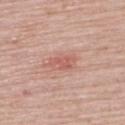Impression: No biopsy was performed on this lesion — it was imaged during a full skin examination and was not determined to be concerning. Acquisition and patient details: A female subject, about 70 years old. From the upper back. The lesion's longest dimension is about 4 mm. Cropped from a total-body skin-imaging series; the visible field is about 15 mm.